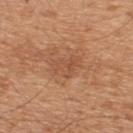follow-up=total-body-photography surveillance lesion; no biopsy | patient=male, about 45 years old | image source=~15 mm crop, total-body skin-cancer survey | site=the upper back.